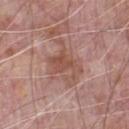Clinical impression: Recorded during total-body skin imaging; not selected for excision or biopsy. Clinical summary: A lesion tile, about 15 mm wide, cut from a 3D total-body photograph. On the chest. A male subject, aged approximately 70.